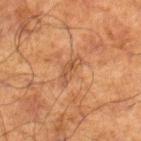Assessment: Captured during whole-body skin photography for melanoma surveillance; the lesion was not biopsied. Context: The lesion is on the right lower leg. The lesion's longest dimension is about 3.5 mm. A 15 mm close-up tile from a total-body photography series done for melanoma screening. Automated image analysis of the tile measured an average lesion color of about L≈43 a*≈19 b*≈31 (CIELAB) and a lesion-to-skin contrast of about 5.5 (normalized; higher = more distinct). A male patient aged around 70.